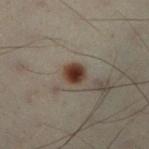Clinical impression:
The lesion was photographed on a routine skin check and not biopsied; there is no pathology result.
Image and clinical context:
A 15 mm close-up extracted from a 3D total-body photography capture. Captured under cross-polarized illumination. An algorithmic analysis of the crop reported a mean CIELAB color near L≈29 a*≈14 b*≈20, a lesion–skin lightness drop of about 12, and a normalized lesion–skin contrast near 13. It also reported a border-irregularity index near 1/10, internal color variation of about 3.5 on a 0–10 scale, and a peripheral color-asymmetry measure near 1. The analysis additionally found a classifier nevus-likeness of about 100/100 and a lesion-detection confidence of about 100/100. A male patient, in their 50s. Longest diameter approximately 2.5 mm. On the left leg.